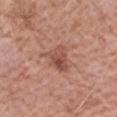<case>
  <biopsy_status>not biopsied; imaged during a skin examination</biopsy_status>
  <patient>
    <sex>female</sex>
    <age_approx>70</age_approx>
  </patient>
  <image>
    <source>total-body photography crop</source>
    <field_of_view_mm>15</field_of_view_mm>
  </image>
  <automated_metrics>
    <area_mm2_approx>7.5</area_mm2_approx>
    <eccentricity>0.5</eccentricity>
    <shape_asymmetry>0.35</shape_asymmetry>
    <cielab_L>51</cielab_L>
    <cielab_a>24</cielab_a>
    <cielab_b>27</cielab_b>
    <vs_skin_darker_L>9.0</vs_skin_darker_L>
    <peripheral_color_asymmetry>2.5</peripheral_color_asymmetry>
  </automated_metrics>
  <site>left forearm</site>
</case>